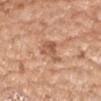Imaged during a routine full-body skin examination; the lesion was not biopsied and no histopathology is available.
Cropped from a total-body skin-imaging series; the visible field is about 15 mm.
Automated image analysis of the tile measured a shape eccentricity near 0.55 and a symmetry-axis asymmetry near 0.45. The software also gave internal color variation of about 4 on a 0–10 scale and radial color variation of about 1.5.
The subject is a female aged around 75.
On the right upper arm.